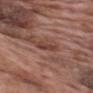biopsy status — imaged on a skin check; not biopsied | diameter — ~2.5 mm (longest diameter) | lighting — white-light | automated metrics — an area of roughly 2.5 mm², a shape eccentricity near 0.85, and a shape-asymmetry score of about 0.55 (0 = symmetric); a mean CIELAB color near L≈41 a*≈24 b*≈27, roughly 8 lightness units darker than nearby skin, and a normalized border contrast of about 7; a nevus-likeness score of about 0/100 | body site — the upper back | acquisition — total-body-photography crop, ~15 mm field of view | subject — male, about 70 years old.